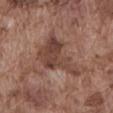{
  "biopsy_status": "not biopsied; imaged during a skin examination"
}